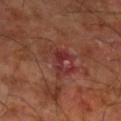<tbp_lesion>
<biopsy_status>not biopsied; imaged during a skin examination</biopsy_status>
<automated_metrics>
  <cielab_L>33</cielab_L>
  <cielab_a>26</cielab_a>
  <cielab_b>22</cielab_b>
  <vs_skin_contrast_norm>6.5</vs_skin_contrast_norm>
  <border_irregularity_0_10>4.5</border_irregularity_0_10>
  <color_variation_0_10>7.5</color_variation_0_10>
  <peripheral_color_asymmetry>2.5</peripheral_color_asymmetry>
</automated_metrics>
<lighting>cross-polarized</lighting>
<site>leg</site>
<image>
  <source>total-body photography crop</source>
  <field_of_view_mm>15</field_of_view_mm>
</image>
<lesion_size>
  <long_diameter_mm_approx>4.5</long_diameter_mm_approx>
</lesion_size>
<patient>
  <sex>male</sex>
  <age_approx>60</age_approx>
</patient>
</tbp_lesion>